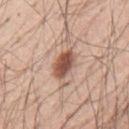Impression: Recorded during total-body skin imaging; not selected for excision or biopsy. Image and clinical context: The recorded lesion diameter is about 3.5 mm. The lesion-visualizer software estimated a footprint of about 7.5 mm². And it measured a lesion color around L≈53 a*≈22 b*≈28 in CIELAB, roughly 16 lightness units darker than nearby skin, and a normalized lesion–skin contrast near 10. And it measured a nevus-likeness score of about 95/100 and a detector confidence of about 100 out of 100 that the crop contains a lesion. From the mid back. The tile uses white-light illumination. A 15 mm close-up extracted from a 3D total-body photography capture. A male patient, aged approximately 55.